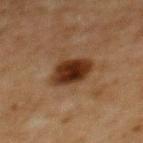{"biopsy_status": "not biopsied; imaged during a skin examination", "image": {"source": "total-body photography crop", "field_of_view_mm": 15}, "lighting": "cross-polarized", "lesion_size": {"long_diameter_mm_approx": 4.0}, "patient": {"sex": "female", "age_approx": 60}, "site": "upper back", "automated_metrics": {"border_irregularity_0_10": 1.5, "color_variation_0_10": 4.5, "peripheral_color_asymmetry": 1.0, "lesion_detection_confidence_0_100": 100}}